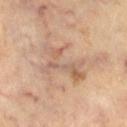{
  "patient": {
    "sex": "female",
    "age_approx": 65
  },
  "image": {
    "source": "total-body photography crop",
    "field_of_view_mm": 15
  },
  "site": "left leg"
}